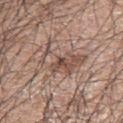A 15 mm crop from a total-body photograph taken for skin-cancer surveillance.
Located on the left upper arm.
A male patient, aged around 70.